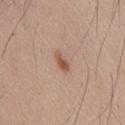The lesion was tiled from a total-body skin photograph and was not biopsied.